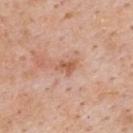This lesion was catalogued during total-body skin photography and was not selected for biopsy. Cropped from a total-body skin-imaging series; the visible field is about 15 mm. From the back. The patient is a male about 60 years old. Longest diameter approximately 3 mm. Imaged with white-light lighting.